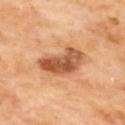<lesion>
<biopsy_status>not biopsied; imaged during a skin examination</biopsy_status>
<lighting>cross-polarized</lighting>
<site>upper back</site>
<image>
  <source>total-body photography crop</source>
  <field_of_view_mm>15</field_of_view_mm>
</image>
<automated_metrics>
  <area_mm2_approx>15.0</area_mm2_approx>
  <eccentricity>0.85</eccentricity>
  <shape_asymmetry>0.35</shape_asymmetry>
  <vs_skin_contrast_norm>9.5</vs_skin_contrast_norm>
</automated_metrics>
</lesion>